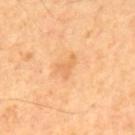Case summary:
– notes: imaged on a skin check; not biopsied
– lesion diameter: about 3 mm
– location: the mid back
– subject: male, aged approximately 65
– imaging modality: ~15 mm crop, total-body skin-cancer survey
– automated lesion analysis: a mean CIELAB color near L≈67 a*≈23 b*≈43, a lesion–skin lightness drop of about 7, and a normalized lesion–skin contrast near 5; a border-irregularity rating of about 7/10 and a peripheral color-asymmetry measure near 0; a classifier nevus-likeness of about 0/100 and lesion-presence confidence of about 100/100